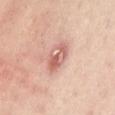Case summary:
– biopsy status — total-body-photography surveillance lesion; no biopsy
– anatomic site — the abdomen
– subject — approximately 55 years of age
– acquisition — ~15 mm tile from a whole-body skin photo